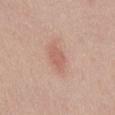biopsy status: no biopsy performed (imaged during a skin exam)
image source: total-body-photography crop, ~15 mm field of view
body site: the front of the torso
patient: male, aged 38 to 42
lesion size: about 3 mm
illumination: white-light